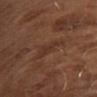Impression:
Recorded during total-body skin imaging; not selected for excision or biopsy.
Acquisition and patient details:
An algorithmic analysis of the crop reported an outline eccentricity of about 0.85 (0 = round, 1 = elongated). The tile uses cross-polarized illumination. The lesion's longest dimension is about 3.5 mm. A 15 mm close-up extracted from a 3D total-body photography capture. A male patient, roughly 65 years of age.